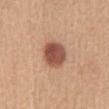Recorded during total-body skin imaging; not selected for excision or biopsy.
The subject is a female about 55 years old.
Automated tile analysis of the lesion measured a lesion color around L≈51 a*≈23 b*≈30 in CIELAB, roughly 16 lightness units darker than nearby skin, and a normalized lesion–skin contrast near 10.5. It also reported a border-irregularity index near 1.5/10, a within-lesion color-variation index near 4.5/10, and radial color variation of about 1.5. And it measured a nevus-likeness score of about 100/100 and a detector confidence of about 100 out of 100 that the crop contains a lesion.
Cropped from a whole-body photographic skin survey; the tile spans about 15 mm.
Captured under white-light illumination.
About 3.5 mm across.
On the abdomen.